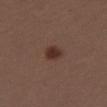Findings:
* site: the right thigh
* image: total-body-photography crop, ~15 mm field of view
* lesion diameter: ≈2.5 mm
* lighting: white-light
* automated metrics: a border-irregularity rating of about 1.5/10, a within-lesion color-variation index near 1.5/10, and peripheral color asymmetry of about 0.5; a classifier nevus-likeness of about 100/100 and lesion-presence confidence of about 100/100
* subject: female, in their 40s